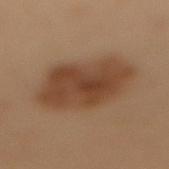Recorded during total-body skin imaging; not selected for excision or biopsy.
About 7.5 mm across.
A female subject aged around 50.
Cropped from a total-body skin-imaging series; the visible field is about 15 mm.
An algorithmic analysis of the crop reported roughly 9 lightness units darker than nearby skin and a lesion-to-skin contrast of about 8.5 (normalized; higher = more distinct). It also reported border irregularity of about 2 on a 0–10 scale, a within-lesion color-variation index near 3.5/10, and peripheral color asymmetry of about 1. The analysis additionally found lesion-presence confidence of about 100/100.
The lesion is located on the mid back.